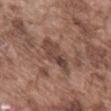Imaged during a routine full-body skin examination; the lesion was not biopsied and no histopathology is available. Located on the abdomen. A male subject, roughly 75 years of age. Automated tile analysis of the lesion measured a footprint of about 10 mm², an eccentricity of roughly 0.85, and two-axis asymmetry of about 0.3. And it measured a lesion–skin lightness drop of about 9. The analysis additionally found border irregularity of about 4 on a 0–10 scale and radial color variation of about 1.5. The software also gave a nevus-likeness score of about 0/100 and a detector confidence of about 80 out of 100 that the crop contains a lesion. A region of skin cropped from a whole-body photographic capture, roughly 15 mm wide. Measured at roughly 5.5 mm in maximum diameter.